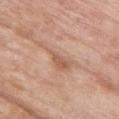Impression:
No biopsy was performed on this lesion — it was imaged during a full skin examination and was not determined to be concerning.
Background:
A roughly 15 mm field-of-view crop from a total-body skin photograph. The patient is a female approximately 70 years of age. From the chest. The recorded lesion diameter is about 6 mm. An algorithmic analysis of the crop reported an outline eccentricity of about 0.95 (0 = round, 1 = elongated) and two-axis asymmetry of about 0.65. It also reported a lesion color around L≈60 a*≈20 b*≈30 in CIELAB, about 7 CIELAB-L* units darker than the surrounding skin, and a normalized lesion–skin contrast near 5. And it measured a classifier nevus-likeness of about 0/100 and a detector confidence of about 100 out of 100 that the crop contains a lesion.